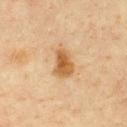Findings:
* biopsy status — total-body-photography surveillance lesion; no biopsy
* imaging modality — total-body-photography crop, ~15 mm field of view
* subject — male, aged 58 to 62
* location — the chest
* automated metrics — an area of roughly 6.5 mm², an eccentricity of roughly 0.8, and a shape-asymmetry score of about 0.25 (0 = symmetric); a lesion–skin lightness drop of about 12; a classifier nevus-likeness of about 95/100